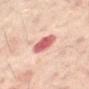workup — catalogued during a skin exam; not biopsied | lesion diameter — ≈3.5 mm | anatomic site — the back | tile lighting — cross-polarized illumination | image-analysis metrics — a footprint of about 7.5 mm², an outline eccentricity of about 0.75 (0 = round, 1 = elongated), and a shape-asymmetry score of about 0.15 (0 = symmetric); a lesion color around L≈58 a*≈30 b*≈24 in CIELAB, a lesion–skin lightness drop of about 15, and a lesion-to-skin contrast of about 9.5 (normalized; higher = more distinct); an automated nevus-likeness rating near 10 out of 100 and lesion-presence confidence of about 100/100 | imaging modality — total-body-photography crop, ~15 mm field of view | subject — male, about 60 years old.